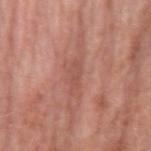Q: Is there a histopathology result?
A: total-body-photography surveillance lesion; no biopsy
Q: What kind of image is this?
A: ~15 mm tile from a whole-body skin photo
Q: How large is the lesion?
A: about 3 mm
Q: What did automated image analysis measure?
A: an area of roughly 3 mm², a shape eccentricity near 0.9, and a symmetry-axis asymmetry near 0.2; an average lesion color of about L≈52 a*≈25 b*≈28 (CIELAB), roughly 6 lightness units darker than nearby skin, and a normalized border contrast of about 4.5; a border-irregularity index near 2.5/10, internal color variation of about 0 on a 0–10 scale, and radial color variation of about 0
Q: What is the anatomic site?
A: the right forearm
Q: What are the patient's age and sex?
A: male, aged around 80
Q: How was the tile lit?
A: white-light illumination